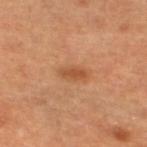{
  "biopsy_status": "not biopsied; imaged during a skin examination",
  "site": "left lower leg",
  "image": {
    "source": "total-body photography crop",
    "field_of_view_mm": 15
  },
  "patient": {
    "sex": "female",
    "age_approx": 60
  }
}